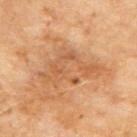Imaged during a routine full-body skin examination; the lesion was not biopsied and no histopathology is available. From the upper back. A roughly 15 mm field-of-view crop from a total-body skin photograph. Automated image analysis of the tile measured a mean CIELAB color near L≈58 a*≈23 b*≈38, a lesion–skin lightness drop of about 8, and a normalized border contrast of about 5.5. The software also gave a within-lesion color-variation index near 4.5/10 and a peripheral color-asymmetry measure near 1.5. And it measured a lesion-detection confidence of about 100/100. A male subject, aged 68 to 72. Longest diameter approximately 7.5 mm.